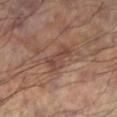Recorded during total-body skin imaging; not selected for excision or biopsy.
A male subject, about 60 years old.
Cropped from a whole-body photographic skin survey; the tile spans about 15 mm.
The lesion is located on the left lower leg.
Captured under cross-polarized illumination.